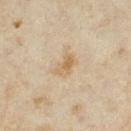Recorded during total-body skin imaging; not selected for excision or biopsy. A 15 mm crop from a total-body photograph taken for skin-cancer surveillance. Longest diameter approximately 3 mm. This is a cross-polarized tile. A female subject, aged approximately 35. From the leg.